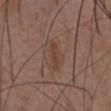A male subject in their 50s. The total-body-photography lesion software estimated a lesion area of about 3.5 mm² and an eccentricity of roughly 0.85. The software also gave a lesion color around L≈41 a*≈18 b*≈26 in CIELAB and a normalized lesion–skin contrast near 6. Cropped from a whole-body photographic skin survey; the tile spans about 15 mm. Located on the chest. Longest diameter approximately 3 mm.